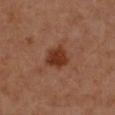Part of a total-body skin-imaging series; this lesion was reviewed on a skin check and was not flagged for biopsy. A lesion tile, about 15 mm wide, cut from a 3D total-body photograph. Longest diameter approximately 3.5 mm. The lesion is on the left forearm. A female patient aged around 50. The lesion-visualizer software estimated a lesion area of about 7.5 mm², an outline eccentricity of about 0.45 (0 = round, 1 = elongated), and two-axis asymmetry of about 0.25. The analysis additionally found a within-lesion color-variation index near 3/10 and peripheral color asymmetry of about 1. The tile uses cross-polarized illumination.